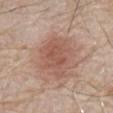{
  "biopsy_status": "not biopsied; imaged during a skin examination",
  "image": {
    "source": "total-body photography crop",
    "field_of_view_mm": 15
  },
  "patient": {
    "sex": "male",
    "age_approx": 80
  },
  "site": "front of the torso"
}